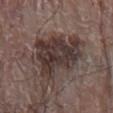This lesion was catalogued during total-body skin photography and was not selected for biopsy. The tile uses white-light illumination. A close-up tile cropped from a whole-body skin photograph, about 15 mm across. The lesion's longest dimension is about 9.5 mm. The subject is a male aged 78–82. The lesion is on the right arm.